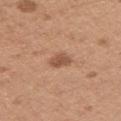Clinical summary: Cropped from a total-body skin-imaging series; the visible field is about 15 mm. The patient is a female aged 28 to 32. Imaged with white-light lighting. The lesion is on the left upper arm. About 2.5 mm across.